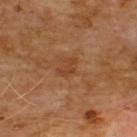Assessment:
This lesion was catalogued during total-body skin photography and was not selected for biopsy.
Background:
A 15 mm close-up extracted from a 3D total-body photography capture. The tile uses cross-polarized illumination. Longest diameter approximately 2.5 mm. A male subject aged around 60. On the chest.